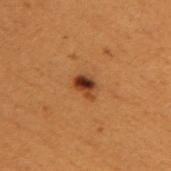Impression:
Recorded during total-body skin imaging; not selected for excision or biopsy.
Acquisition and patient details:
Imaged with cross-polarized lighting. The lesion-visualizer software estimated a footprint of about 3.5 mm² and a shape eccentricity near 0.7. Cropped from a whole-body photographic skin survey; the tile spans about 15 mm. Located on the upper back. The lesion's longest dimension is about 2.5 mm. The patient is a male in their 50s.